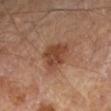Recorded during total-body skin imaging; not selected for excision or biopsy. A male subject aged around 65. This is a cross-polarized tile. Automated image analysis of the tile measured a lesion color around L≈42 a*≈21 b*≈29 in CIELAB, roughly 10 lightness units darker than nearby skin, and a lesion-to-skin contrast of about 8 (normalized; higher = more distinct). The software also gave a border-irregularity index near 2.5/10 and a peripheral color-asymmetry measure near 1.5. Measured at roughly 4 mm in maximum diameter. A 15 mm close-up tile from a total-body photography series done for melanoma screening. From the right forearm.